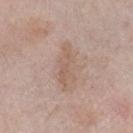anatomic site = the right lower leg | illumination = white-light | acquisition = ~15 mm crop, total-body skin-cancer survey | subject = female, aged around 65 | diameter = about 4.5 mm.